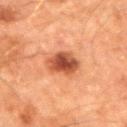notes: no biopsy performed (imaged during a skin exam)